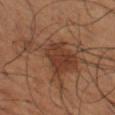follow-up: no biopsy performed (imaged during a skin exam) | image: total-body-photography crop, ~15 mm field of view | automated metrics: a mean CIELAB color near L≈40 a*≈20 b*≈29 and a normalized border contrast of about 7; a classifier nevus-likeness of about 70/100 and a detector confidence of about 100 out of 100 that the crop contains a lesion | site: the left thigh | illumination: cross-polarized | patient: male, in their mid-60s | lesion diameter: ≈8.5 mm.